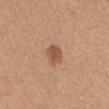Assessment:
Recorded during total-body skin imaging; not selected for excision or biopsy.
Acquisition and patient details:
About 2.5 mm across. A lesion tile, about 15 mm wide, cut from a 3D total-body photograph. The subject is a female in their mid-50s. Imaged with white-light lighting. The lesion is located on the front of the torso.